This image is a 15 mm lesion crop taken from a total-body photograph; a male patient roughly 40 years of age; on the abdomen: 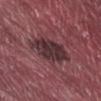Diagnosis:
Histopathology of the biopsied lesion showed a dysplastic (Clark) nevus.